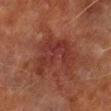| key | value |
|---|---|
| biopsy status | catalogued during a skin exam; not biopsied |
| illumination | cross-polarized |
| TBP lesion metrics | an average lesion color of about L≈28 a*≈23 b*≈23 (CIELAB), a lesion–skin lightness drop of about 6, and a lesion-to-skin contrast of about 7 (normalized; higher = more distinct); a color-variation rating of about 3.5/10 and a peripheral color-asymmetry measure near 1; a nevus-likeness score of about 0/100 and a lesion-detection confidence of about 100/100 |
| image | total-body-photography crop, ~15 mm field of view |
| site | the right lower leg |
| lesion diameter | ~6.5 mm (longest diameter) |
| subject | male, in their 70s |